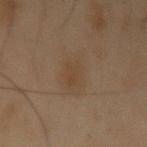notes = imaged on a skin check; not biopsied | image = ~15 mm crop, total-body skin-cancer survey | location = the left upper arm | subject = male, about 55 years old.